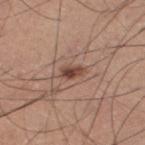Q: Was this lesion biopsied?
A: total-body-photography surveillance lesion; no biopsy
Q: What is the anatomic site?
A: the right thigh
Q: Who is the patient?
A: male, about 55 years old
Q: What kind of image is this?
A: ~15 mm tile from a whole-body skin photo